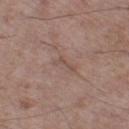Case summary:
- follow-up · catalogued during a skin exam; not biopsied
- patient · male, approximately 50 years of age
- illumination · white-light
- diameter · ≈3 mm
- location · the left thigh
- image source · ~15 mm tile from a whole-body skin photo